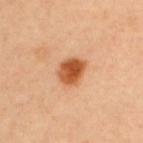| key | value |
|---|---|
| lighting | cross-polarized illumination |
| location | the upper back |
| acquisition | ~15 mm tile from a whole-body skin photo |
| patient | male, aged 43 to 47 |
| image-analysis metrics | a footprint of about 7.5 mm² and an outline eccentricity of about 0.6 (0 = round, 1 = elongated); a border-irregularity index near 1.5/10 and radial color variation of about 1.5; an automated nevus-likeness rating near 100 out of 100 and a lesion-detection confidence of about 100/100 |